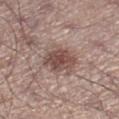follow-up: total-body-photography surveillance lesion; no biopsy | image source: ~15 mm tile from a whole-body skin photo | subject: male, in their mid-50s | illumination: white-light illumination | site: the right lower leg | image-analysis metrics: a border-irregularity index near 3/10 and a color-variation rating of about 4/10; a nevus-likeness score of about 90/100 and a lesion-detection confidence of about 100/100 | lesion diameter: ~4.5 mm (longest diameter).